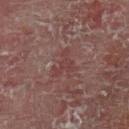This lesion was catalogued during total-body skin photography and was not selected for biopsy.
The lesion's longest dimension is about 3.5 mm.
The lesion is located on the left lower leg.
A lesion tile, about 15 mm wide, cut from a 3D total-body photograph.
The subject is a male aged 58–62.
This is a cross-polarized tile.